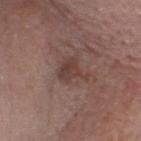Clinical impression: The lesion was tiled from a total-body skin photograph and was not biopsied. Image and clinical context: A 15 mm close-up tile from a total-body photography series done for melanoma screening. The lesion is on the head or neck. Longest diameter approximately 3.5 mm. A male subject, aged 68 to 72.